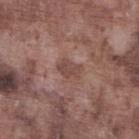biopsy_status: not biopsied; imaged during a skin examination
patient:
  sex: male
  age_approx: 75
image:
  source: total-body photography crop
  field_of_view_mm: 15
site: left lower leg